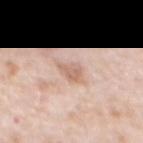biopsy_status: not biopsied; imaged during a skin examination
patient:
  sex: male
  age_approx: 80
automated_metrics:
  area_mm2_approx: 4.0
  eccentricity: 0.5
  shape_asymmetry: 0.4
image:
  source: total-body photography crop
  field_of_view_mm: 15
lesion_size:
  long_diameter_mm_approx: 2.5
lighting: white-light
site: front of the torso